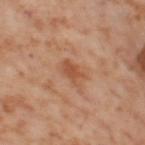Recorded during total-body skin imaging; not selected for excision or biopsy.
A 15 mm close-up tile from a total-body photography series done for melanoma screening.
Imaged with cross-polarized lighting.
Measured at roughly 3.5 mm in maximum diameter.
A female patient, aged 53–57.
The lesion is located on the left thigh.